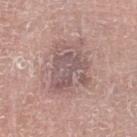The lesion was tiled from a total-body skin photograph and was not biopsied. Approximately 5.5 mm at its widest. The patient is a male aged 73–77. The lesion is located on the left lower leg. Cropped from a whole-body photographic skin survey; the tile spans about 15 mm. Automated tile analysis of the lesion measured a footprint of about 17 mm², an eccentricity of roughly 0.6, and a shape-asymmetry score of about 0.4 (0 = symmetric). It also reported a mean CIELAB color near L≈55 a*≈17 b*≈19, about 9 CIELAB-L* units darker than the surrounding skin, and a normalized lesion–skin contrast near 6.5. And it measured border irregularity of about 5.5 on a 0–10 scale, a color-variation rating of about 4.5/10, and radial color variation of about 1.5. It also reported an automated nevus-likeness rating near 0 out of 100 and a detector confidence of about 100 out of 100 that the crop contains a lesion.